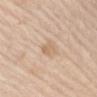Imaged during a routine full-body skin examination; the lesion was not biopsied and no histopathology is available. The lesion-visualizer software estimated a footprint of about 3 mm², an outline eccentricity of about 0.85 (0 = round, 1 = elongated), and two-axis asymmetry of about 0.3. The software also gave a detector confidence of about 100 out of 100 that the crop contains a lesion. A 15 mm close-up tile from a total-body photography series done for melanoma screening. The lesion is located on the right upper arm. The recorded lesion diameter is about 2.5 mm. A male subject, aged approximately 65. Imaged with white-light lighting.